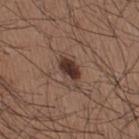The lesion is on the right thigh.
The tile uses white-light illumination.
A 15 mm close-up extracted from a 3D total-body photography capture.
The lesion-visualizer software estimated a lesion area of about 7 mm², a shape eccentricity near 0.85, and a symmetry-axis asymmetry near 0.25. And it measured a border-irregularity index near 3/10 and a color-variation rating of about 4/10.
A male patient about 35 years old.
The recorded lesion diameter is about 4.5 mm.